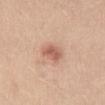Q: Was this lesion biopsied?
A: no biopsy performed (imaged during a skin exam)
Q: Lesion location?
A: the abdomen
Q: What lighting was used for the tile?
A: white-light illumination
Q: How was this image acquired?
A: ~15 mm crop, total-body skin-cancer survey
Q: Lesion size?
A: ~3 mm (longest diameter)
Q: Patient demographics?
A: female, aged approximately 25